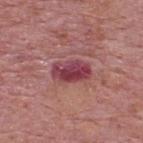Assessment: No biopsy was performed on this lesion — it was imaged during a full skin examination and was not determined to be concerning. Acquisition and patient details: A male subject aged approximately 75. The lesion is located on the back. A region of skin cropped from a whole-body photographic capture, roughly 15 mm wide. Approximately 4.5 mm at its widest. The total-body-photography lesion software estimated an area of roughly 10 mm², an outline eccentricity of about 0.8 (0 = round, 1 = elongated), and a shape-asymmetry score of about 0.15 (0 = symmetric). The analysis additionally found a within-lesion color-variation index near 6/10 and peripheral color asymmetry of about 2. And it measured a nevus-likeness score of about 30/100. Captured under white-light illumination.